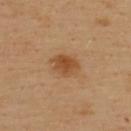Assessment: The lesion was photographed on a routine skin check and not biopsied; there is no pathology result. Context: A 15 mm crop from a total-body photograph taken for skin-cancer surveillance. A male patient about 40 years old. Located on the upper back.